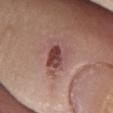Imaged during a routine full-body skin examination; the lesion was not biopsied and no histopathology is available.
The tile uses white-light illumination.
The lesion is located on the leg.
A roughly 15 mm field-of-view crop from a total-body skin photograph.
Automated image analysis of the tile measured border irregularity of about 3 on a 0–10 scale, a color-variation rating of about 7/10, and a peripheral color-asymmetry measure near 2.
The recorded lesion diameter is about 3.5 mm.
The subject is a female aged around 75.